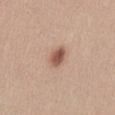This lesion was catalogued during total-body skin photography and was not selected for biopsy. Located on the abdomen. A close-up tile cropped from a whole-body skin photograph, about 15 mm across. Approximately 3 mm at its widest. A female patient aged 18 to 22. This is a white-light tile. Automated tile analysis of the lesion measured a border-irregularity rating of about 1.5/10, a within-lesion color-variation index near 3.5/10, and a peripheral color-asymmetry measure near 1.5.